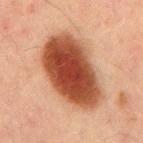<lesion>
  <image>
    <source>total-body photography crop</source>
    <field_of_view_mm>15</field_of_view_mm>
  </image>
  <lesion_size>
    <long_diameter_mm_approx>9.0</long_diameter_mm_approx>
  </lesion_size>
  <patient>
    <sex>male</sex>
    <age_approx>50</age_approx>
  </patient>
  <site>mid back</site>
  <lighting>cross-polarized</lighting>
</lesion>